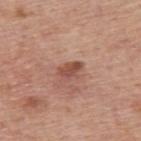The lesion is located on the upper back.
The recorded lesion diameter is about 3 mm.
The subject is a male aged approximately 75.
This is a white-light tile.
Cropped from a whole-body photographic skin survey; the tile spans about 15 mm.